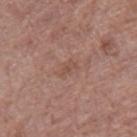Clinical impression:
Part of a total-body skin-imaging series; this lesion was reviewed on a skin check and was not flagged for biopsy.
Context:
An algorithmic analysis of the crop reported a lesion–skin lightness drop of about 6 and a normalized border contrast of about 4.5. It also reported a border-irregularity rating of about 3.5/10, internal color variation of about 2 on a 0–10 scale, and radial color variation of about 0.5. And it measured a classifier nevus-likeness of about 0/100 and a lesion-detection confidence of about 100/100. The recorded lesion diameter is about 2.5 mm. The tile uses white-light illumination. A 15 mm crop from a total-body photograph taken for skin-cancer surveillance. Located on the right thigh. The patient is a female aged 73 to 77.